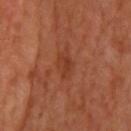Impression: The lesion was photographed on a routine skin check and not biopsied; there is no pathology result. Image and clinical context: A 15 mm close-up tile from a total-body photography series done for melanoma screening. Imaged with cross-polarized lighting. A male patient aged around 65. From the head or neck.